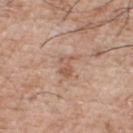No biopsy was performed on this lesion — it was imaged during a full skin examination and was not determined to be concerning. The patient is a male in their mid-70s. The lesion is located on the front of the torso. Longest diameter approximately 2.5 mm. Captured under white-light illumination. A 15 mm close-up tile from a total-body photography series done for melanoma screening.